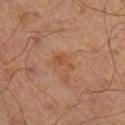| field | value |
|---|---|
| notes | imaged on a skin check; not biopsied |
| size | about 3 mm |
| acquisition | total-body-photography crop, ~15 mm field of view |
| illumination | cross-polarized illumination |
| body site | the leg |
| subject | male, in their mid- to late 60s |